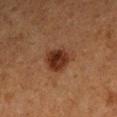follow-up: total-body-photography surveillance lesion; no biopsy
site: the left forearm
diameter: about 4 mm
patient: female, aged 38 to 42
lighting: cross-polarized
acquisition: ~15 mm tile from a whole-body skin photo